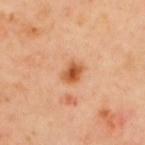Case summary:
* notes: catalogued during a skin exam; not biopsied